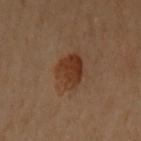Findings:
• site: the upper back
• patient: female, about 60 years old
• tile lighting: cross-polarized
• image source: total-body-photography crop, ~15 mm field of view
• image-analysis metrics: a lesion area of about 9.5 mm² and two-axis asymmetry of about 0.15; an average lesion color of about L≈30 a*≈18 b*≈27 (CIELAB), a lesion–skin lightness drop of about 8, and a normalized lesion–skin contrast near 8.5; border irregularity of about 1.5 on a 0–10 scale, a color-variation rating of about 3.5/10, and peripheral color asymmetry of about 1.5
• lesion size: about 3.5 mm